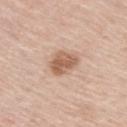<case>
<biopsy_status>not biopsied; imaged during a skin examination</biopsy_status>
<patient>
  <sex>male</sex>
  <age_approx>60</age_approx>
</patient>
<automated_metrics>
  <eccentricity>0.35</eccentricity>
  <shape_asymmetry>0.3</shape_asymmetry>
  <border_irregularity_0_10>2.5</border_irregularity_0_10>
  <color_variation_0_10>3.5</color_variation_0_10>
  <peripheral_color_asymmetry>1.0</peripheral_color_asymmetry>
  <nevus_likeness_0_100>55</nevus_likeness_0_100>
</automated_metrics>
<lighting>white-light</lighting>
<lesion_size>
  <long_diameter_mm_approx>3.0</long_diameter_mm_approx>
</lesion_size>
<site>upper back</site>
<image>
  <source>total-body photography crop</source>
  <field_of_view_mm>15</field_of_view_mm>
</image>
</case>